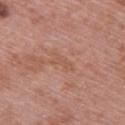Imaged during a routine full-body skin examination; the lesion was not biopsied and no histopathology is available. Measured at roughly 3 mm in maximum diameter. Imaged with white-light lighting. The lesion is located on the upper back. The subject is a female aged 58–62. Automated image analysis of the tile measured a lesion area of about 3 mm², an outline eccentricity of about 0.9 (0 = round, 1 = elongated), and a symmetry-axis asymmetry near 0.4. It also reported a mean CIELAB color near L≈54 a*≈23 b*≈31, about 5 CIELAB-L* units darker than the surrounding skin, and a normalized border contrast of about 5. The software also gave a detector confidence of about 95 out of 100 that the crop contains a lesion. A 15 mm close-up tile from a total-body photography series done for melanoma screening.